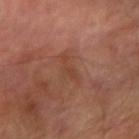The lesion was photographed on a routine skin check and not biopsied; there is no pathology result.
The total-body-photography lesion software estimated a lesion color around L≈44 a*≈24 b*≈31 in CIELAB, about 6 CIELAB-L* units darker than the surrounding skin, and a normalized lesion–skin contrast near 5. It also reported a border-irregularity rating of about 6/10, internal color variation of about 0.5 on a 0–10 scale, and peripheral color asymmetry of about 0. The software also gave a classifier nevus-likeness of about 0/100.
A roughly 15 mm field-of-view crop from a total-body skin photograph.
Longest diameter approximately 3.5 mm.
The lesion is on the right forearm.
Imaged with cross-polarized lighting.